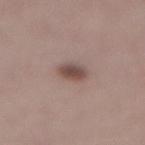  biopsy_status: not biopsied; imaged during a skin examination
  automated_metrics:
    area_mm2_approx: 5.0
    eccentricity: 0.3
    shape_asymmetry: 0.2
    border_irregularity_0_10: 1.5
    color_variation_0_10: 4.0
    peripheral_color_asymmetry: 1.0
    nevus_likeness_0_100: 95
    lesion_detection_confidence_0_100: 100
  patient:
    sex: female
    age_approx: 45
  image:
    source: total-body photography crop
    field_of_view_mm: 15
  site: lower back
  lighting: white-light